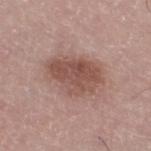<record>
  <biopsy_status>not biopsied; imaged during a skin examination</biopsy_status>
  <site>right thigh</site>
  <patient>
    <sex>male</sex>
    <age_approx>65</age_approx>
  </patient>
  <image>
    <source>total-body photography crop</source>
    <field_of_view_mm>15</field_of_view_mm>
  </image>
  <automated_metrics>
    <area_mm2_approx>18.0</area_mm2_approx>
    <eccentricity>0.75</eccentricity>
    <shape_asymmetry>0.2</shape_asymmetry>
    <border_irregularity_0_10>3.0</border_irregularity_0_10>
    <nevus_likeness_0_100>50</nevus_likeness_0_100>
    <lesion_detection_confidence_0_100>100</lesion_detection_confidence_0_100>
  </automated_metrics>
  <lighting>white-light</lighting>
  <lesion_size>
    <long_diameter_mm_approx>6.0</long_diameter_mm_approx>
  </lesion_size>
</record>